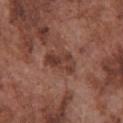Clinical impression:
Part of a total-body skin-imaging series; this lesion was reviewed on a skin check and was not flagged for biopsy.
Context:
Approximately 4 mm at its widest. From the front of the torso. The total-body-photography lesion software estimated a mean CIELAB color near L≈38 a*≈22 b*≈25, roughly 9 lightness units darker than nearby skin, and a normalized lesion–skin contrast near 7.5. It also reported a border-irregularity rating of about 3/10. And it measured a nevus-likeness score of about 10/100. A region of skin cropped from a whole-body photographic capture, roughly 15 mm wide. The tile uses white-light illumination. The patient is a male in their mid- to late 70s.